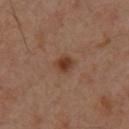workup = imaged on a skin check; not biopsied | site = the upper back | diameter = ≈2 mm | patient = male, roughly 55 years of age | image = 15 mm crop, total-body photography | tile lighting = cross-polarized illumination.